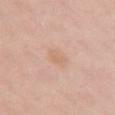<tbp_lesion>
<biopsy_status>not biopsied; imaged during a skin examination</biopsy_status>
<lesion_size>
  <long_diameter_mm_approx>2.5</long_diameter_mm_approx>
</lesion_size>
<image>
  <source>total-body photography crop</source>
  <field_of_view_mm>15</field_of_view_mm>
</image>
<patient>
  <sex>female</sex>
  <age_approx>35</age_approx>
</patient>
<site>chest</site>
</tbp_lesion>